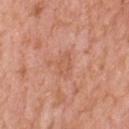Impression: Part of a total-body skin-imaging series; this lesion was reviewed on a skin check and was not flagged for biopsy. Background: The patient is a female roughly 70 years of age. From the back. A roughly 15 mm field-of-view crop from a total-body skin photograph.